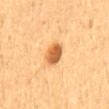{"biopsy_status": "not biopsied; imaged during a skin examination", "site": "abdomen", "patient": {"sex": "male", "age_approx": 45}, "automated_metrics": {"eccentricity": 0.55, "shape_asymmetry": 0.15, "cielab_L": 59, "cielab_a": 25, "cielab_b": 44, "vs_skin_darker_L": 15.0, "color_variation_0_10": 3.5}, "image": {"source": "total-body photography crop", "field_of_view_mm": 15}, "lighting": "cross-polarized", "lesion_size": {"long_diameter_mm_approx": 3.0}}